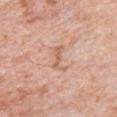Part of a total-body skin-imaging series; this lesion was reviewed on a skin check and was not flagged for biopsy. A male subject, roughly 80 years of age. Captured under white-light illumination. Automated image analysis of the tile measured a mean CIELAB color near L≈63 a*≈22 b*≈31, roughly 8 lightness units darker than nearby skin, and a normalized border contrast of about 5.5. A roughly 15 mm field-of-view crop from a total-body skin photograph. Longest diameter approximately 3.5 mm. The lesion is located on the chest.